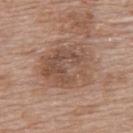  biopsy_status: not biopsied; imaged during a skin examination
  site: upper back
  patient:
    sex: female
    age_approx: 60
  lesion_size:
    long_diameter_mm_approx: 5.5
  image:
    source: total-body photography crop
    field_of_view_mm: 15